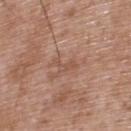biopsy status = imaged on a skin check; not biopsied
location = the upper back
image = 15 mm crop, total-body photography
patient = male, about 50 years old